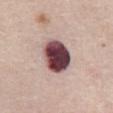Clinical impression:
This lesion was catalogued during total-body skin photography and was not selected for biopsy.
Image and clinical context:
The lesion is located on the abdomen. An algorithmic analysis of the crop reported a lesion area of about 15 mm² and a shape eccentricity near 0.45. The software also gave a border-irregularity rating of about 1/10. The patient is a female aged approximately 70. Longest diameter approximately 4 mm. A 15 mm close-up extracted from a 3D total-body photography capture.